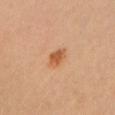workup: imaged on a skin check; not biopsied | subject: female, aged 33–37 | site: the head or neck | image: 15 mm crop, total-body photography.